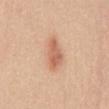Impression: Imaged during a routine full-body skin examination; the lesion was not biopsied and no histopathology is available. Context: The lesion's longest dimension is about 4 mm. On the front of the torso. Captured under white-light illumination. The total-body-photography lesion software estimated an area of roughly 7 mm² and two-axis asymmetry of about 0.25. It also reported an average lesion color of about L≈62 a*≈24 b*≈33 (CIELAB), about 11 CIELAB-L* units darker than the surrounding skin, and a normalized border contrast of about 7. This image is a 15 mm lesion crop taken from a total-body photograph. A female subject approximately 20 years of age.